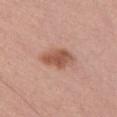Impression:
This lesion was catalogued during total-body skin photography and was not selected for biopsy.
Clinical summary:
A 15 mm crop from a total-body photograph taken for skin-cancer surveillance. Located on the chest. Measured at roughly 5 mm in maximum diameter. Automated tile analysis of the lesion measured a border-irregularity rating of about 2/10 and radial color variation of about 1. Captured under white-light illumination. A female patient, in their mid-30s.